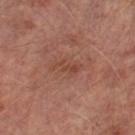Notes:
– workup · total-body-photography surveillance lesion; no biopsy
– anatomic site · the left lower leg
– patient · male, aged 63–67
– tile lighting · cross-polarized
– imaging modality · ~15 mm crop, total-body skin-cancer survey
– automated lesion analysis · a footprint of about 3.5 mm², an outline eccentricity of about 0.9 (0 = round, 1 = elongated), and a symmetry-axis asymmetry near 0.5